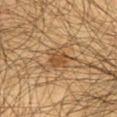<lesion>
  <biopsy_status>not biopsied; imaged during a skin examination</biopsy_status>
  <image>
    <source>total-body photography crop</source>
    <field_of_view_mm>15</field_of_view_mm>
  </image>
  <patient>
    <sex>male</sex>
    <age_approx>60</age_approx>
  </patient>
  <lesion_size>
    <long_diameter_mm_approx>3.5</long_diameter_mm_approx>
  </lesion_size>
  <lighting>cross-polarized</lighting>
  <site>front of the torso</site>
</lesion>